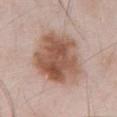Q: Was a biopsy performed?
A: catalogued during a skin exam; not biopsied
Q: Who is the patient?
A: male, about 55 years old
Q: Lesion location?
A: the abdomen
Q: What kind of image is this?
A: 15 mm crop, total-body photography
Q: Lesion size?
A: ~7.5 mm (longest diameter)